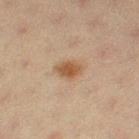Captured during whole-body skin photography for melanoma surveillance; the lesion was not biopsied. From the left thigh. An algorithmic analysis of the crop reported a footprint of about 5.5 mm², an outline eccentricity of about 0.75 (0 = round, 1 = elongated), and a symmetry-axis asymmetry near 0.25. The software also gave an average lesion color of about L≈47 a*≈16 b*≈31 (CIELAB), about 9 CIELAB-L* units darker than the surrounding skin, and a normalized lesion–skin contrast near 8. The software also gave a color-variation rating of about 2.5/10 and peripheral color asymmetry of about 0.5. The analysis additionally found a nevus-likeness score of about 90/100. Imaged with cross-polarized lighting. A female patient, aged 18–22. A 15 mm close-up extracted from a 3D total-body photography capture. The recorded lesion diameter is about 3.5 mm.